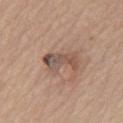Q: Is there a histopathology result?
A: catalogued during a skin exam; not biopsied
Q: What is the anatomic site?
A: the chest
Q: What is the lesion's diameter?
A: ~4.5 mm (longest diameter)
Q: What is the imaging modality?
A: total-body-photography crop, ~15 mm field of view
Q: What are the patient's age and sex?
A: male, roughly 80 years of age
Q: What lighting was used for the tile?
A: white-light illumination